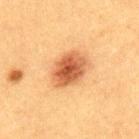Assessment:
Imaged during a routine full-body skin examination; the lesion was not biopsied and no histopathology is available.
Clinical summary:
A 15 mm crop from a total-body photograph taken for skin-cancer surveillance. About 5 mm across. A male patient aged around 50. The lesion is located on the back. An algorithmic analysis of the crop reported an area of roughly 14 mm². It also reported a lesion-detection confidence of about 100/100.